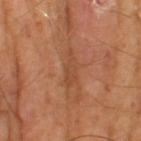Imaged during a routine full-body skin examination; the lesion was not biopsied and no histopathology is available.
A lesion tile, about 15 mm wide, cut from a 3D total-body photograph.
The lesion's longest dimension is about 4 mm.
The patient is a male roughly 65 years of age.
Automated tile analysis of the lesion measured a lesion area of about 5 mm², an eccentricity of roughly 0.95, and two-axis asymmetry of about 0.35. The analysis additionally found a mean CIELAB color near L≈46 a*≈23 b*≈34, about 7 CIELAB-L* units darker than the surrounding skin, and a normalized border contrast of about 5.5. The software also gave a nevus-likeness score of about 0/100 and a detector confidence of about 75 out of 100 that the crop contains a lesion.